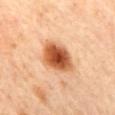| field | value |
|---|---|
| workup | catalogued during a skin exam; not biopsied |
| acquisition | total-body-photography crop, ~15 mm field of view |
| patient | male, roughly 55 years of age |
| location | the mid back |